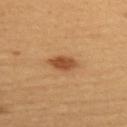Q: Is there a histopathology result?
A: catalogued during a skin exam; not biopsied
Q: How was this image acquired?
A: ~15 mm tile from a whole-body skin photo
Q: Lesion location?
A: the upper back
Q: How was the tile lit?
A: cross-polarized
Q: Patient demographics?
A: female, approximately 40 years of age
Q: How large is the lesion?
A: ≈3.5 mm